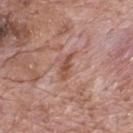Image and clinical context: Cropped from a whole-body photographic skin survey; the tile spans about 15 mm. A male subject, approximately 70 years of age. The lesion is located on the mid back. An algorithmic analysis of the crop reported an area of roughly 3 mm² and a shape eccentricity near 0.9. The analysis additionally found a lesion color around L≈51 a*≈23 b*≈28 in CIELAB, about 10 CIELAB-L* units darker than the surrounding skin, and a lesion-to-skin contrast of about 7 (normalized; higher = more distinct). It also reported border irregularity of about 3.5 on a 0–10 scale, a color-variation rating of about 1/10, and a peripheral color-asymmetry measure near 0. The analysis additionally found an automated nevus-likeness rating near 0 out of 100 and a detector confidence of about 100 out of 100 that the crop contains a lesion. This is a white-light tile.